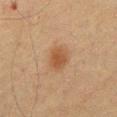notes: no biopsy performed (imaged during a skin exam).